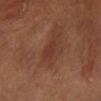notes: imaged on a skin check; not biopsied | subject: male, aged 53–57 | lesion diameter: ≈3 mm | image: total-body-photography crop, ~15 mm field of view | location: the left lower leg.